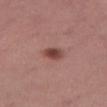Assessment: Recorded during total-body skin imaging; not selected for excision or biopsy. Clinical summary: The recorded lesion diameter is about 3 mm. A 15 mm crop from a total-body photograph taken for skin-cancer surveillance. The lesion-visualizer software estimated an outline eccentricity of about 0.65 (0 = round, 1 = elongated) and two-axis asymmetry of about 0.2. And it measured a mean CIELAB color near L≈45 a*≈23 b*≈24, a lesion–skin lightness drop of about 12, and a normalized border contrast of about 8.5. It also reported a border-irregularity index near 1.5/10, a color-variation rating of about 4.5/10, and peripheral color asymmetry of about 1.5. From the right thigh. Captured under white-light illumination. A female patient approximately 55 years of age.